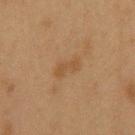follow-up = total-body-photography surveillance lesion; no biopsy
image = 15 mm crop, total-body photography
size = ~3 mm (longest diameter)
illumination = cross-polarized illumination
body site = the chest
patient = male, in their mid- to late 50s